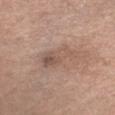follow-up = no biopsy performed (imaged during a skin exam)
acquisition = ~15 mm tile from a whole-body skin photo
lesion size = ≈5 mm
subject = female, about 65 years old
site = the chest
tile lighting = white-light illumination
TBP lesion metrics = a lesion color around L≈54 a*≈17 b*≈25 in CIELAB, roughly 8 lightness units darker than nearby skin, and a normalized border contrast of about 5.5; a border-irregularity index near 4.5/10, a within-lesion color-variation index near 5.5/10, and a peripheral color-asymmetry measure near 2; an automated nevus-likeness rating near 0 out of 100 and a detector confidence of about 100 out of 100 that the crop contains a lesion